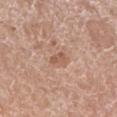Recorded during total-body skin imaging; not selected for excision or biopsy.
The subject is a female about 75 years old.
The lesion is located on the left lower leg.
This image is a 15 mm lesion crop taken from a total-body photograph.